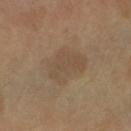Case summary:
– notes · total-body-photography surveillance lesion; no biopsy
– body site · the left arm
– acquisition · ~15 mm crop, total-body skin-cancer survey
– tile lighting · cross-polarized
– patient · female, aged 58 to 62
– lesion size · about 4.5 mm